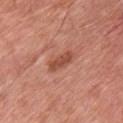Clinical impression:
The lesion was tiled from a total-body skin photograph and was not biopsied.
Clinical summary:
The lesion is on the chest. Cropped from a whole-body photographic skin survey; the tile spans about 15 mm. A male patient aged 58 to 62. About 3.5 mm across. The lesion-visualizer software estimated an area of roughly 5 mm², a shape eccentricity near 0.9, and a symmetry-axis asymmetry near 0.2. And it measured a nevus-likeness score of about 35/100. This is a white-light tile.